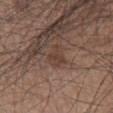This lesion was catalogued during total-body skin photography and was not selected for biopsy.
The lesion is located on the leg.
A roughly 15 mm field-of-view crop from a total-body skin photograph.
Imaged with white-light lighting.
A male subject, approximately 45 years of age.